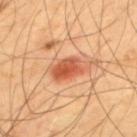Impression: Recorded during total-body skin imaging; not selected for excision or biopsy. Acquisition and patient details: This is a cross-polarized tile. The lesion is on the mid back. A lesion tile, about 15 mm wide, cut from a 3D total-body photograph. A male subject aged 63 to 67. Approximately 5 mm at its widest.